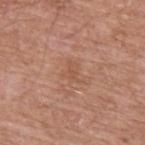Imaged during a routine full-body skin examination; the lesion was not biopsied and no histopathology is available. A male subject, aged approximately 60. The tile uses white-light illumination. The recorded lesion diameter is about 2.5 mm. The lesion-visualizer software estimated an average lesion color of about L≈53 a*≈23 b*≈32 (CIELAB). The analysis additionally found an automated nevus-likeness rating near 0 out of 100 and a detector confidence of about 100 out of 100 that the crop contains a lesion. On the left upper arm. A 15 mm close-up extracted from a 3D total-body photography capture.